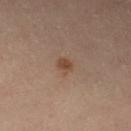biopsy status = total-body-photography surveillance lesion; no biopsy
body site = the left thigh
image = 15 mm crop, total-body photography
diameter = ≈2 mm
subject = female, in their mid- to late 50s
illumination = cross-polarized illumination
automated metrics = a footprint of about 2.5 mm² and a shape eccentricity near 0.55; a border-irregularity rating of about 2/10, internal color variation of about 1.5 on a 0–10 scale, and radial color variation of about 0.5